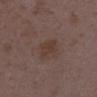Impression:
Imaged during a routine full-body skin examination; the lesion was not biopsied and no histopathology is available.
Acquisition and patient details:
On the left lower leg. A female patient, about 35 years old. Imaged with white-light lighting. This image is a 15 mm lesion crop taken from a total-body photograph.